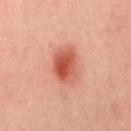Clinical impression:
Part of a total-body skin-imaging series; this lesion was reviewed on a skin check and was not flagged for biopsy.
Clinical summary:
A 15 mm close-up extracted from a 3D total-body photography capture. A male patient, in their 40s. From the mid back.